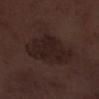Part of a total-body skin-imaging series; this lesion was reviewed on a skin check and was not flagged for biopsy. From the leg. A roughly 15 mm field-of-view crop from a total-body skin photograph. A male subject in their 70s. The tile uses white-light illumination. The lesion's longest dimension is about 7 mm.